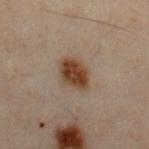biopsy status: imaged on a skin check; not biopsied
patient: male, about 50 years old
acquisition: ~15 mm tile from a whole-body skin photo
body site: the chest